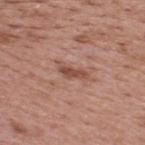Clinical impression: This lesion was catalogued during total-body skin photography and was not selected for biopsy.